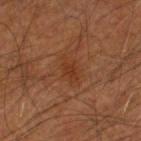Captured during whole-body skin photography for melanoma surveillance; the lesion was not biopsied. Approximately 3.5 mm at its widest. A male patient, aged around 60. A region of skin cropped from a whole-body photographic capture, roughly 15 mm wide. On the head or neck. This is a cross-polarized tile. An algorithmic analysis of the crop reported a lesion area of about 5 mm², an outline eccentricity of about 0.8 (0 = round, 1 = elongated), and a symmetry-axis asymmetry near 0.25. The analysis additionally found a mean CIELAB color near L≈33 a*≈22 b*≈31, roughly 5 lightness units darker than nearby skin, and a normalized lesion–skin contrast near 5.5. And it measured a detector confidence of about 100 out of 100 that the crop contains a lesion.